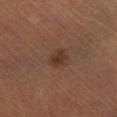No biopsy was performed on this lesion — it was imaged during a full skin examination and was not determined to be concerning.
Measured at roughly 2.5 mm in maximum diameter.
A roughly 15 mm field-of-view crop from a total-body skin photograph.
A male subject, in their 50s.
Captured under cross-polarized illumination.
The lesion is on the right lower leg.